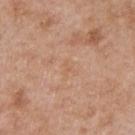Context: The recorded lesion diameter is about 1.5 mm. The tile uses white-light illumination. A 15 mm close-up tile from a total-body photography series done for melanoma screening. The patient is a male about 50 years old. The lesion is located on the chest.